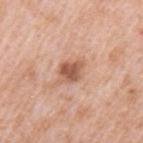Notes:
- follow-up · catalogued during a skin exam; not biopsied